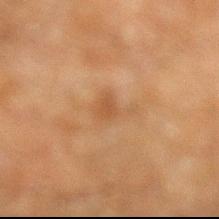Imaged during a routine full-body skin examination; the lesion was not biopsied and no histopathology is available. The patient is a male aged around 60. The lesion is located on the left lower leg. Approximately 3.5 mm at its widest. The tile uses cross-polarized illumination. A 15 mm close-up tile from a total-body photography series done for melanoma screening.